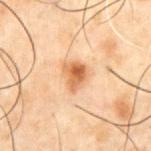Recorded during total-body skin imaging; not selected for excision or biopsy.
On the abdomen.
Imaged with cross-polarized lighting.
A male patient, approximately 50 years of age.
A close-up tile cropped from a whole-body skin photograph, about 15 mm across.